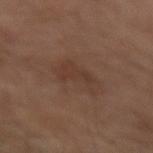  biopsy_status: not biopsied; imaged during a skin examination
  image:
    source: total-body photography crop
    field_of_view_mm: 15
  patient:
    sex: male
    age_approx: 65
  site: arm